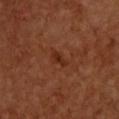The lesion was photographed on a routine skin check and not biopsied; there is no pathology result.
Automated image analysis of the tile measured roughly 6 lightness units darker than nearby skin and a lesion-to-skin contrast of about 6.5 (normalized; higher = more distinct).
The lesion is located on the upper back.
The subject is a male aged 58 to 62.
This is a cross-polarized tile.
A lesion tile, about 15 mm wide, cut from a 3D total-body photograph.
About 3 mm across.